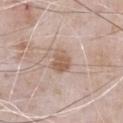notes: no biopsy performed (imaged during a skin exam) | automated metrics: an average lesion color of about L≈58 a*≈17 b*≈28 (CIELAB) and a lesion–skin lightness drop of about 10; a color-variation rating of about 3/10 and a peripheral color-asymmetry measure near 1; an automated nevus-likeness rating near 30 out of 100 and a detector confidence of about 100 out of 100 that the crop contains a lesion | anatomic site: the chest | lesion size: ~3 mm (longest diameter) | subject: male, roughly 50 years of age | image: 15 mm crop, total-body photography.